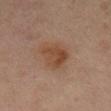follow-up: catalogued during a skin exam; not biopsied
patient: female, in their 70s
image: total-body-photography crop, ~15 mm field of view
lighting: cross-polarized illumination
site: the right lower leg
size: about 4.5 mm